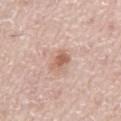lesion size: ~3 mm (longest diameter) | TBP lesion metrics: a footprint of about 4 mm², a shape eccentricity near 0.8, and a shape-asymmetry score of about 0.25 (0 = symmetric); roughly 11 lightness units darker than nearby skin; a color-variation rating of about 2.5/10 and radial color variation of about 1; lesion-presence confidence of about 100/100 | location: the abdomen | illumination: white-light | acquisition: 15 mm crop, total-body photography | patient: male, in their mid-70s.